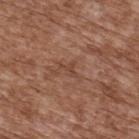follow-up: total-body-photography surveillance lesion; no biopsy
anatomic site: the upper back
patient: male, in their mid- to late 70s
lighting: white-light illumination
imaging modality: total-body-photography crop, ~15 mm field of view
size: ≈2.5 mm